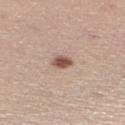| key | value |
|---|---|
| follow-up | total-body-photography surveillance lesion; no biopsy |
| image source | total-body-photography crop, ~15 mm field of view |
| subject | female, aged 58–62 |
| location | the right lower leg |
| tile lighting | white-light illumination |